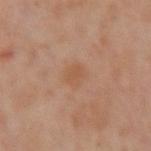The lesion was photographed on a routine skin check and not biopsied; there is no pathology result. A female subject aged 38–42. This image is a 15 mm lesion crop taken from a total-body photograph. The lesion is on the left arm.